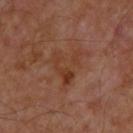biopsy status = total-body-photography surveillance lesion; no biopsy | lesion diameter = ~5 mm (longest diameter) | subject = male, aged 53 to 57 | image-analysis metrics = a footprint of about 11 mm², a shape eccentricity near 0.5, and two-axis asymmetry of about 0.65; a mean CIELAB color near L≈37 a*≈22 b*≈29, about 6 CIELAB-L* units darker than the surrounding skin, and a normalized border contrast of about 6; an automated nevus-likeness rating near 0 out of 100 | lighting = cross-polarized | body site = the upper back | image = 15 mm crop, total-body photography.